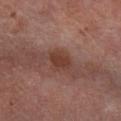This lesion was catalogued during total-body skin photography and was not selected for biopsy.
Cropped from a total-body skin-imaging series; the visible field is about 15 mm.
From the right lower leg.
The patient is a male roughly 60 years of age.
The lesion's longest dimension is about 3 mm.
Captured under cross-polarized illumination.